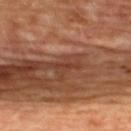A female subject aged around 45. The lesion is on the upper back. Approximately 3 mm at its widest. This is a cross-polarized tile. A 15 mm close-up tile from a total-body photography series done for melanoma screening. The total-body-photography lesion software estimated a lesion color around L≈37 a*≈23 b*≈30 in CIELAB, about 7 CIELAB-L* units darker than the surrounding skin, and a normalized border contrast of about 6.5. And it measured border irregularity of about 5.5 on a 0–10 scale, internal color variation of about 0 on a 0–10 scale, and peripheral color asymmetry of about 0. The software also gave a classifier nevus-likeness of about 0/100 and lesion-presence confidence of about 55/100.